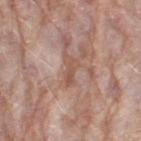No biopsy was performed on this lesion — it was imaged during a full skin examination and was not determined to be concerning.
The patient is a female roughly 70 years of age.
Located on the leg.
A region of skin cropped from a whole-body photographic capture, roughly 15 mm wide.